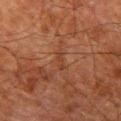Part of a total-body skin-imaging series; this lesion was reviewed on a skin check and was not flagged for biopsy. A male patient aged 78 to 82. The lesion-visualizer software estimated a border-irregularity index near 4/10, internal color variation of about 0 on a 0–10 scale, and a peripheral color-asymmetry measure near 0. A region of skin cropped from a whole-body photographic capture, roughly 15 mm wide. From the leg.